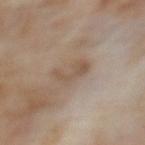Notes:
– workup: imaged on a skin check; not biopsied
– subject: female, aged approximately 60
– lesion diameter: ≈4 mm
– lighting: cross-polarized
– imaging modality: total-body-photography crop, ~15 mm field of view
– location: the upper back